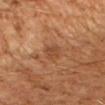Captured during whole-body skin photography for melanoma surveillance; the lesion was not biopsied. From the right forearm. A male patient, about 60 years old. The recorded lesion diameter is about 3 mm. The lesion-visualizer software estimated a mean CIELAB color near L≈43 a*≈22 b*≈32, roughly 7 lightness units darker than nearby skin, and a normalized border contrast of about 5.5. Imaged with cross-polarized lighting. A region of skin cropped from a whole-body photographic capture, roughly 15 mm wide.